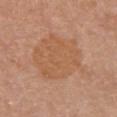| field | value |
|---|---|
| workup | catalogued during a skin exam; not biopsied |
| acquisition | ~15 mm tile from a whole-body skin photo |
| size | about 8 mm |
| site | the chest |
| subject | female, aged 53 to 57 |